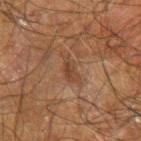Q: Was a biopsy performed?
A: imaged on a skin check; not biopsied
Q: Illumination type?
A: cross-polarized
Q: What is the anatomic site?
A: the right forearm
Q: What did automated image analysis measure?
A: a shape-asymmetry score of about 0.3 (0 = symmetric); a border-irregularity rating of about 3/10, internal color variation of about 2 on a 0–10 scale, and a peripheral color-asymmetry measure near 1
Q: What is the imaging modality?
A: 15 mm crop, total-body photography
Q: Who is the patient?
A: male, aged around 70
Q: Lesion size?
A: about 3 mm